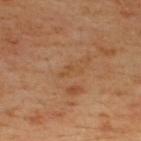The lesion was photographed on a routine skin check and not biopsied; there is no pathology result. A lesion tile, about 15 mm wide, cut from a 3D total-body photograph. Automated image analysis of the tile measured a lesion area of about 2.5 mm², a shape eccentricity near 0.9, and a shape-asymmetry score of about 0.5 (0 = symmetric). The tile uses cross-polarized illumination. Measured at roughly 3 mm in maximum diameter. A male patient in their mid- to late 40s. From the upper back.